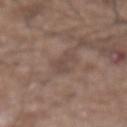workup = total-body-photography surveillance lesion; no biopsy
lesion diameter = ~3 mm (longest diameter)
site = the abdomen
image source = ~15 mm crop, total-body skin-cancer survey
subject = male, roughly 75 years of age
tile lighting = white-light illumination